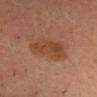No biopsy was performed on this lesion — it was imaged during a full skin examination and was not determined to be concerning. The tile uses cross-polarized illumination. A region of skin cropped from a whole-body photographic capture, roughly 15 mm wide. From the head or neck. Automated image analysis of the tile measured an average lesion color of about L≈38 a*≈19 b*≈29 (CIELAB), about 7 CIELAB-L* units darker than the surrounding skin, and a lesion-to-skin contrast of about 7 (normalized; higher = more distinct). And it measured an automated nevus-likeness rating near 10 out of 100 and lesion-presence confidence of about 100/100. The recorded lesion diameter is about 5.5 mm. A male subject, in their mid-30s.